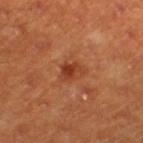A male patient aged around 60.
Captured under cross-polarized illumination.
A roughly 15 mm field-of-view crop from a total-body skin photograph.
From the left thigh.